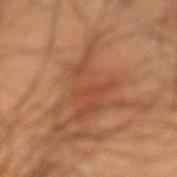Impression:
Imaged during a routine full-body skin examination; the lesion was not biopsied and no histopathology is available.
Context:
Imaged with cross-polarized lighting. This image is a 15 mm lesion crop taken from a total-body photograph. The lesion is on the abdomen. Measured at roughly 7.5 mm in maximum diameter. A male patient approximately 55 years of age.